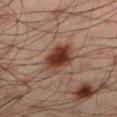Case summary:
– biopsy status — imaged on a skin check; not biopsied
– patient — male, aged 33–37
– size — ~4 mm (longest diameter)
– image-analysis metrics — an average lesion color of about L≈35 a*≈20 b*≈26 (CIELAB), roughly 13 lightness units darker than nearby skin, and a normalized border contrast of about 11; a border-irregularity index near 1.5/10 and a peripheral color-asymmetry measure near 1.5
– image source — 15 mm crop, total-body photography
– location — the left lower leg
– illumination — cross-polarized illumination